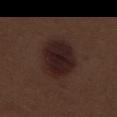Q: Was this lesion biopsied?
A: catalogued during a skin exam; not biopsied
Q: Illumination type?
A: white-light
Q: Automated lesion metrics?
A: a mean CIELAB color near L≈20 a*≈16 b*≈16 and a lesion–skin lightness drop of about 9; a color-variation rating of about 3.5/10 and a peripheral color-asymmetry measure near 1
Q: What are the patient's age and sex?
A: male, aged 68 to 72
Q: How was this image acquired?
A: ~15 mm crop, total-body skin-cancer survey
Q: What is the anatomic site?
A: the left thigh